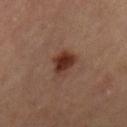* workup · total-body-photography surveillance lesion; no biopsy
* tile lighting · cross-polarized
* acquisition · 15 mm crop, total-body photography
* anatomic site · the right lower leg
* automated metrics · a lesion area of about 6.5 mm² and an eccentricity of roughly 0.5; a lesion-to-skin contrast of about 11 (normalized; higher = more distinct); border irregularity of about 2 on a 0–10 scale, a within-lesion color-variation index near 4/10, and radial color variation of about 1
* patient · female, aged around 65